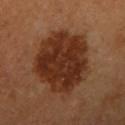This lesion was catalogued during total-body skin photography and was not selected for biopsy.
A female subject, in their 50s.
A 15 mm close-up tile from a total-body photography series done for melanoma screening.
Approximately 7.5 mm at its widest.
Imaged with cross-polarized lighting.
From the right upper arm.
Automated tile analysis of the lesion measured about 12 CIELAB-L* units darker than the surrounding skin and a normalized lesion–skin contrast near 11. The software also gave an automated nevus-likeness rating near 100 out of 100 and lesion-presence confidence of about 100/100.